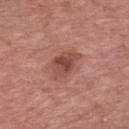<case>
  <biopsy_status>not biopsied; imaged during a skin examination</biopsy_status>
  <image>
    <source>total-body photography crop</source>
    <field_of_view_mm>15</field_of_view_mm>
  </image>
  <site>chest</site>
  <lesion_size>
    <long_diameter_mm_approx>4.0</long_diameter_mm_approx>
  </lesion_size>
  <patient>
    <sex>male</sex>
    <age_approx>75</age_approx>
  </patient>
</case>